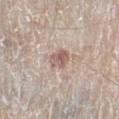| field | value |
|---|---|
| notes | catalogued during a skin exam; not biopsied |
| imaging modality | 15 mm crop, total-body photography |
| automated lesion analysis | an area of roughly 5 mm², an outline eccentricity of about 0.7 (0 = round, 1 = elongated), and a symmetry-axis asymmetry near 0.2; an automated nevus-likeness rating near 0 out of 100 |
| subject | male, aged approximately 80 |
| location | the left lower leg |
| diameter | ~3 mm (longest diameter) |
| illumination | white-light |